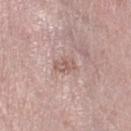Assessment:
Recorded during total-body skin imaging; not selected for excision or biopsy.
Acquisition and patient details:
A 15 mm close-up tile from a total-body photography series done for melanoma screening. A female patient in their 70s. This is a white-light tile. The lesion is on the right lower leg.